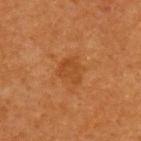Impression:
The lesion was photographed on a routine skin check and not biopsied; there is no pathology result.
Clinical summary:
A male patient roughly 60 years of age. On the back. A 15 mm close-up tile from a total-body photography series done for melanoma screening. The lesion-visualizer software estimated a lesion area of about 6.5 mm² and an outline eccentricity of about 0.5 (0 = round, 1 = elongated). The analysis additionally found a mean CIELAB color near L≈44 a*≈26 b*≈41, a lesion–skin lightness drop of about 6, and a normalized lesion–skin contrast near 5.5. It also reported a border-irregularity index near 3.5/10 and peripheral color asymmetry of about 0.5.